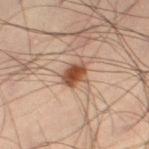  biopsy_status: not biopsied; imaged during a skin examination
  image:
    source: total-body photography crop
    field_of_view_mm: 15
  site: leg
  patient:
    sex: male
    age_approx: 40
  lighting: cross-polarized